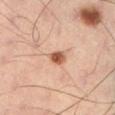No biopsy was performed on this lesion — it was imaged during a full skin examination and was not determined to be concerning. The tile uses cross-polarized illumination. The lesion's longest dimension is about 2.5 mm. On the left lower leg. Cropped from a whole-body photographic skin survey; the tile spans about 15 mm. A male patient, aged 38 to 42.